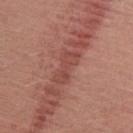size: ≈4 mm | site: the right upper arm | automated lesion analysis: a lesion color around L≈47 a*≈27 b*≈26 in CIELAB, a lesion–skin lightness drop of about 8, and a lesion-to-skin contrast of about 6 (normalized; higher = more distinct) | imaging modality: total-body-photography crop, ~15 mm field of view | subject: female, aged approximately 50.